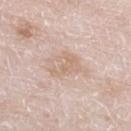Impression:
Imaged during a routine full-body skin examination; the lesion was not biopsied and no histopathology is available.
Context:
The lesion is on the left lower leg. A male subject aged 78–82. A 15 mm close-up tile from a total-body photography series done for melanoma screening.